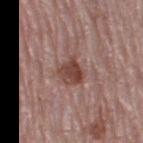Part of a total-body skin-imaging series; this lesion was reviewed on a skin check and was not flagged for biopsy. A roughly 15 mm field-of-view crop from a total-body skin photograph. Captured under white-light illumination. A female patient about 65 years old. The lesion's longest dimension is about 3.5 mm. The lesion is located on the right thigh.